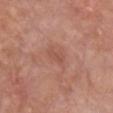automated lesion analysis = a lesion area of about 3 mm², a shape eccentricity near 0.85, and two-axis asymmetry of about 0.2; a border-irregularity rating of about 2.5/10, a within-lesion color-variation index near 0/10, and radial color variation of about 0
illumination = white-light
site = the chest
lesion size = ~2.5 mm (longest diameter)
patient = male, approximately 80 years of age
image = 15 mm crop, total-body photography